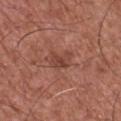subject=male, aged around 75; tile lighting=white-light illumination; image-analysis metrics=a classifier nevus-likeness of about 10/100 and lesion-presence confidence of about 100/100; diameter=~3 mm (longest diameter); image source=~15 mm tile from a whole-body skin photo; site=the chest.